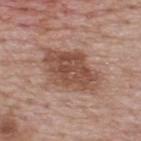Image and clinical context: Automated image analysis of the tile measured a lesion area of about 22 mm². And it measured an average lesion color of about L≈50 a*≈21 b*≈27 (CIELAB) and a lesion-to-skin contrast of about 8.5 (normalized; higher = more distinct). And it measured a classifier nevus-likeness of about 80/100 and a lesion-detection confidence of about 100/100. From the upper back. A close-up tile cropped from a whole-body skin photograph, about 15 mm across. This is a white-light tile. Approximately 7 mm at its widest. A male patient, in their mid-60s.